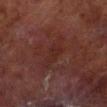Clinical impression:
Recorded during total-body skin imaging; not selected for excision or biopsy.
Context:
A lesion tile, about 15 mm wide, cut from a 3D total-body photograph. The lesion is located on the leg. This is a cross-polarized tile. Automated tile analysis of the lesion measured a lesion area of about 6.5 mm² and two-axis asymmetry of about 0.45. And it measured a lesion color around L≈21 a*≈19 b*≈19 in CIELAB and a normalized lesion–skin contrast near 5. It also reported a border-irregularity rating of about 6/10 and a within-lesion color-variation index near 2/10. A male subject, about 70 years old.